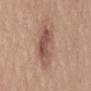Findings:
– follow-up · imaged on a skin check; not biopsied
– anatomic site · the lower back
– image · ~15 mm tile from a whole-body skin photo
– lighting · white-light illumination
– size · about 4.5 mm
– subject · female, about 20 years old
– image-analysis metrics · a mean CIELAB color near L≈52 a*≈20 b*≈26, a lesion–skin lightness drop of about 11, and a normalized border contrast of about 7.5